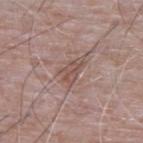Q: Was this lesion biopsied?
A: total-body-photography surveillance lesion; no biopsy
Q: How large is the lesion?
A: about 4 mm
Q: What kind of image is this?
A: ~15 mm tile from a whole-body skin photo
Q: What lighting was used for the tile?
A: white-light
Q: What is the anatomic site?
A: the upper back
Q: What are the patient's age and sex?
A: male, aged 63 to 67